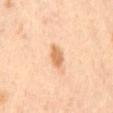patient = female, aged approximately 55
location = the mid back
lesion diameter = ≈3 mm
automated lesion analysis = a lesion area of about 4.5 mm², an eccentricity of roughly 0.8, and a symmetry-axis asymmetry near 0.2; a nevus-likeness score of about 90/100 and a detector confidence of about 100 out of 100 that the crop contains a lesion
image source = ~15 mm tile from a whole-body skin photo
illumination = cross-polarized illumination